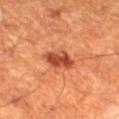{"biopsy_status": "not biopsied; imaged during a skin examination", "image": {"source": "total-body photography crop", "field_of_view_mm": 15}, "lighting": "cross-polarized", "automated_metrics": {"vs_skin_darker_L": 12.0, "vs_skin_contrast_norm": 9.0, "nevus_likeness_0_100": 90, "lesion_detection_confidence_0_100": 100}, "site": "leg", "patient": {"sex": "male", "age_approx": 60}}